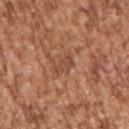Q: Was a biopsy performed?
A: no biopsy performed (imaged during a skin exam)
Q: Patient demographics?
A: male, in their mid-40s
Q: What kind of image is this?
A: total-body-photography crop, ~15 mm field of view
Q: What is the anatomic site?
A: the arm